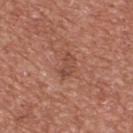| field | value |
|---|---|
| image-analysis metrics | border irregularity of about 6.5 on a 0–10 scale, a color-variation rating of about 0/10, and radial color variation of about 0 |
| image source | total-body-photography crop, ~15 mm field of view |
| location | the chest |
| patient | male, roughly 45 years of age |
| lighting | white-light |
| lesion diameter | ≈3 mm |